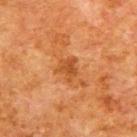workup: total-body-photography surveillance lesion; no biopsy
image source: 15 mm crop, total-body photography
anatomic site: the upper back
lesion diameter: about 3 mm
subject: male, in their 80s
automated metrics: a footprint of about 5 mm², an outline eccentricity of about 0.6 (0 = round, 1 = elongated), and a symmetry-axis asymmetry near 0.45; border irregularity of about 4.5 on a 0–10 scale; a nevus-likeness score of about 10/100 and lesion-presence confidence of about 100/100
illumination: cross-polarized illumination